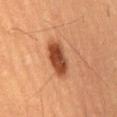{"biopsy_status": "not biopsied; imaged during a skin examination", "image": {"source": "total-body photography crop", "field_of_view_mm": 15}, "patient": {"sex": "male", "age_approx": 55}, "site": "lower back"}